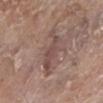This lesion was catalogued during total-body skin photography and was not selected for biopsy. A female subject aged approximately 85 or older. The tile uses white-light illumination. Cropped from a total-body skin-imaging series; the visible field is about 15 mm. The lesion is located on the left lower leg.